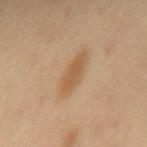follow-up — total-body-photography surveillance lesion; no biopsy | size — ~6 mm (longest diameter) | patient — female, in their 60s | automated metrics — a footprint of about 8.5 mm², a shape eccentricity near 0.95, and a shape-asymmetry score of about 0.2 (0 = symmetric); a border-irregularity rating of about 3.5/10 and a color-variation rating of about 2/10 | location — the mid back | tile lighting — cross-polarized | image — ~15 mm crop, total-body skin-cancer survey.